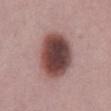Part of a total-body skin-imaging series; this lesion was reviewed on a skin check and was not flagged for biopsy. A male patient aged around 50. From the abdomen. A lesion tile, about 15 mm wide, cut from a 3D total-body photograph.